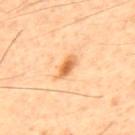Captured during whole-body skin photography for melanoma surveillance; the lesion was not biopsied.
Measured at roughly 3.5 mm in maximum diameter.
Imaged with cross-polarized lighting.
The lesion is on the back.
Cropped from a whole-body photographic skin survey; the tile spans about 15 mm.
Automated image analysis of the tile measured a classifier nevus-likeness of about 95/100 and a lesion-detection confidence of about 100/100.
A male subject, aged 58 to 62.